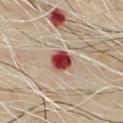Notes:
– biopsy status: catalogued during a skin exam; not biopsied
– subject: male, roughly 55 years of age
– location: the chest
– lighting: cross-polarized illumination
– diameter: ≈2.5 mm
– imaging modality: ~15 mm crop, total-body skin-cancer survey